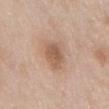Assessment:
The lesion was tiled from a total-body skin photograph and was not biopsied.
Acquisition and patient details:
Automated tile analysis of the lesion measured a within-lesion color-variation index near 3/10 and a peripheral color-asymmetry measure near 1. The lesion is on the abdomen. A roughly 15 mm field-of-view crop from a total-body skin photograph. This is a white-light tile. A male patient aged around 65. Measured at roughly 3.5 mm in maximum diameter.